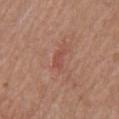subject: male, aged 63 to 67 | acquisition: ~15 mm tile from a whole-body skin photo | diameter: ≈2.5 mm | lighting: white-light illumination | body site: the front of the torso | TBP lesion metrics: a footprint of about 3 mm², an eccentricity of roughly 0.85, and a shape-asymmetry score of about 0.4 (0 = symmetric); roughly 7 lightness units darker than nearby skin and a normalized lesion–skin contrast near 5; a border-irregularity index near 4/10, a color-variation rating of about 0.5/10, and radial color variation of about 0.